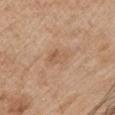Findings:
- subject — male, aged approximately 65
- automated metrics — a lesion area of about 4 mm², a shape eccentricity near 0.7, and a shape-asymmetry score of about 0.25 (0 = symmetric); a border-irregularity rating of about 2/10 and a color-variation rating of about 3.5/10
- tile lighting — white-light
- acquisition — 15 mm crop, total-body photography
- lesion diameter — ~2.5 mm (longest diameter)
- anatomic site — the front of the torso